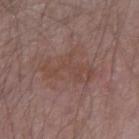follow-up: total-body-photography surveillance lesion; no biopsy.